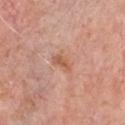follow-up: total-body-photography surveillance lesion; no biopsy | body site: the chest | patient: male, roughly 80 years of age | image source: 15 mm crop, total-body photography.